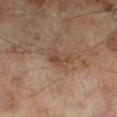Context:
The tile uses cross-polarized illumination. The patient is a male about 65 years old. Automated tile analysis of the lesion measured an area of roughly 4 mm², a shape eccentricity near 0.75, and two-axis asymmetry of about 0.25. And it measured an average lesion color of about L≈43 a*≈18 b*≈26 (CIELAB) and roughly 7 lightness units darker than nearby skin. And it measured a border-irregularity index near 2.5/10, a color-variation rating of about 3.5/10, and a peripheral color-asymmetry measure near 1. And it measured a classifier nevus-likeness of about 0/100 and a lesion-detection confidence of about 100/100. From the left lower leg. A 15 mm close-up extracted from a 3D total-body photography capture. Measured at roughly 3 mm in maximum diameter.